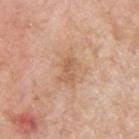Imaged during a routine full-body skin examination; the lesion was not biopsied and no histopathology is available.
The patient is a male roughly 60 years of age.
The lesion-visualizer software estimated a border-irregularity index near 5/10, a color-variation rating of about 0/10, and a peripheral color-asymmetry measure near 0.
Captured under white-light illumination.
A lesion tile, about 15 mm wide, cut from a 3D total-body photograph.
The lesion is on the upper back.
About 3 mm across.